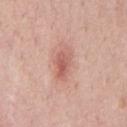Captured during whole-body skin photography for melanoma surveillance; the lesion was not biopsied. This image is a 15 mm lesion crop taken from a total-body photograph. A male patient, aged around 55. Located on the chest.